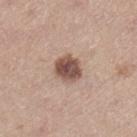Case summary:
• notes · total-body-photography surveillance lesion; no biopsy
• size · about 3 mm
• imaging modality · 15 mm crop, total-body photography
• image-analysis metrics · a lesion area of about 7.5 mm², an eccentricity of roughly 0.4, and a symmetry-axis asymmetry near 0.1; about 16 CIELAB-L* units darker than the surrounding skin and a lesion-to-skin contrast of about 11 (normalized; higher = more distinct); a border-irregularity index near 1/10, internal color variation of about 5 on a 0–10 scale, and a peripheral color-asymmetry measure near 1.5
• subject · female, in their mid-40s
• tile lighting · white-light illumination
• location · the right lower leg